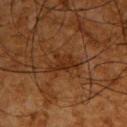Impression:
The lesion was photographed on a routine skin check and not biopsied; there is no pathology result.
Acquisition and patient details:
On the back. Imaged with cross-polarized lighting. The patient is a male aged 63 to 67. About 4.5 mm across. Automated image analysis of the tile measured a footprint of about 7 mm² and two-axis asymmetry of about 0.45. The analysis additionally found a mean CIELAB color near L≈24 a*≈18 b*≈28, a lesion–skin lightness drop of about 6, and a lesion-to-skin contrast of about 6.5 (normalized; higher = more distinct). The software also gave border irregularity of about 6 on a 0–10 scale and a within-lesion color-variation index near 1.5/10. And it measured a nevus-likeness score of about 0/100 and lesion-presence confidence of about 55/100. Cropped from a whole-body photographic skin survey; the tile spans about 15 mm.